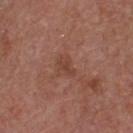follow-up=no biopsy performed (imaged during a skin exam)
diameter=~2.5 mm (longest diameter)
automated lesion analysis=a border-irregularity index near 4/10 and a peripheral color-asymmetry measure near 0; a nevus-likeness score of about 0/100 and a detector confidence of about 100 out of 100 that the crop contains a lesion
subject=male, approximately 55 years of age
body site=the chest
image source=~15 mm crop, total-body skin-cancer survey
tile lighting=white-light